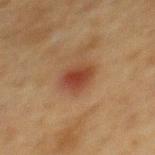The tile uses cross-polarized illumination.
The lesion is located on the mid back.
A male patient, aged 73 to 77.
A 15 mm close-up tile from a total-body photography series done for melanoma screening.
The recorded lesion diameter is about 3.5 mm.
Automated image analysis of the tile measured a footprint of about 8 mm² and a shape eccentricity near 0.5. And it measured a border-irregularity index near 1.5/10 and internal color variation of about 4 on a 0–10 scale. And it measured a nevus-likeness score of about 95/100 and a lesion-detection confidence of about 100/100.